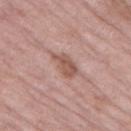{"biopsy_status": "not biopsied; imaged during a skin examination", "automated_metrics": {"shape_asymmetry": 0.3, "vs_skin_darker_L": 10.0, "vs_skin_contrast_norm": 7.0, "nevus_likeness_0_100": 0, "lesion_detection_confidence_0_100": 100}, "lighting": "white-light", "patient": {"sex": "female", "age_approx": 70}, "site": "left thigh", "lesion_size": {"long_diameter_mm_approx": 4.0}, "image": {"source": "total-body photography crop", "field_of_view_mm": 15}}